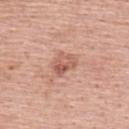Q: Patient demographics?
A: male, in their 60s
Q: What kind of image is this?
A: 15 mm crop, total-body photography
Q: How was the tile lit?
A: white-light
Q: What is the anatomic site?
A: the upper back
Q: How large is the lesion?
A: ~3 mm (longest diameter)